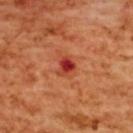follow-up: catalogued during a skin exam; not biopsied
patient: female, approximately 55 years of age
imaging modality: total-body-photography crop, ~15 mm field of view
anatomic site: the upper back
automated lesion analysis: an average lesion color of about L≈43 a*≈40 b*≈37 (CIELAB), about 13 CIELAB-L* units darker than the surrounding skin, and a normalized lesion–skin contrast near 9.5; an automated nevus-likeness rating near 0 out of 100